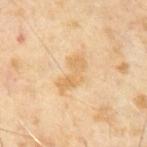Impression:
The lesion was photographed on a routine skin check and not biopsied; there is no pathology result.
Image and clinical context:
A male subject in their mid- to late 60s. A 15 mm close-up tile from a total-body photography series done for melanoma screening.